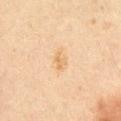Acquisition and patient details: Captured under cross-polarized illumination. A male patient about 60 years old. This image is a 15 mm lesion crop taken from a total-body photograph. Measured at roughly 3 mm in maximum diameter. On the abdomen.